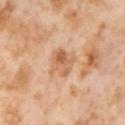Part of a total-body skin-imaging series; this lesion was reviewed on a skin check and was not flagged for biopsy. The total-body-photography lesion software estimated a lesion area of about 7.5 mm² and an outline eccentricity of about 0.55 (0 = round, 1 = elongated). The software also gave a lesion-detection confidence of about 100/100. A female subject aged 53 to 57. A roughly 15 mm field-of-view crop from a total-body skin photograph. The lesion is on the right thigh. Captured under cross-polarized illumination. The lesion's longest dimension is about 3.5 mm.